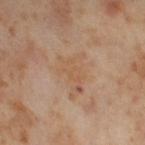Acquisition and patient details:
A female subject, aged approximately 55. Automated image analysis of the tile measured a shape-asymmetry score of about 0.5 (0 = symmetric). The analysis additionally found a lesion color around L≈57 a*≈18 b*≈32 in CIELAB and a normalized lesion–skin contrast near 4.5. It also reported a border-irregularity rating of about 6/10, a within-lesion color-variation index near 2.5/10, and radial color variation of about 1. The lesion is located on the right thigh. A 15 mm close-up extracted from a 3D total-body photography capture. Longest diameter approximately 5 mm.